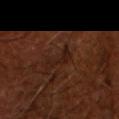{
  "biopsy_status": "not biopsied; imaged during a skin examination",
  "image": {
    "source": "total-body photography crop",
    "field_of_view_mm": 15
  },
  "lighting": "cross-polarized",
  "patient": {
    "sex": "male",
    "age_approx": 65
  },
  "automated_metrics": {
    "area_mm2_approx": 4.0,
    "eccentricity": 0.9,
    "shape_asymmetry": 0.5,
    "nevus_likeness_0_100": 0,
    "lesion_detection_confidence_0_100": 55
  }
}